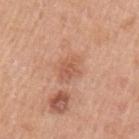No biopsy was performed on this lesion — it was imaged during a full skin examination and was not determined to be concerning.